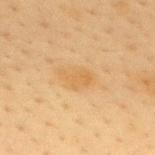Captured during whole-body skin photography for melanoma surveillance; the lesion was not biopsied. A female patient roughly 40 years of age. About 4 mm across. The lesion is located on the back. Automated tile analysis of the lesion measured an area of roughly 7.5 mm² and a symmetry-axis asymmetry near 0.2. It also reported a border-irregularity index near 2.5/10, internal color variation of about 2 on a 0–10 scale, and peripheral color asymmetry of about 0.5. The analysis additionally found a classifier nevus-likeness of about 5/100 and a lesion-detection confidence of about 100/100. A roughly 15 mm field-of-view crop from a total-body skin photograph.